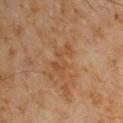A male subject, aged around 60. The lesion is on the arm. A roughly 15 mm field-of-view crop from a total-body skin photograph.